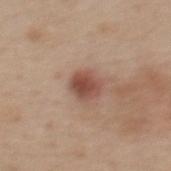Clinical impression: This lesion was catalogued during total-body skin photography and was not selected for biopsy. Background: The lesion is on the mid back. This is a white-light tile. A roughly 15 mm field-of-view crop from a total-body skin photograph. About 3 mm across. An algorithmic analysis of the crop reported a lesion color around L≈49 a*≈22 b*≈27 in CIELAB and about 12 CIELAB-L* units darker than the surrounding skin. The analysis additionally found a border-irregularity index near 2/10 and radial color variation of about 1.5. The software also gave a nevus-likeness score of about 95/100 and lesion-presence confidence of about 100/100. A male subject, aged 58–62.